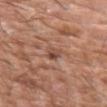Imaged during a routine full-body skin examination; the lesion was not biopsied and no histopathology is available.
From the left upper arm.
Longest diameter approximately 2.5 mm.
A male patient about 75 years old.
Captured under white-light illumination.
A 15 mm close-up tile from a total-body photography series done for melanoma screening.